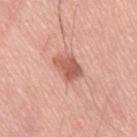Q: Was this lesion biopsied?
A: total-body-photography surveillance lesion; no biopsy
Q: What is the lesion's diameter?
A: about 3.5 mm
Q: What lighting was used for the tile?
A: white-light illumination
Q: How was this image acquired?
A: ~15 mm crop, total-body skin-cancer survey
Q: Where on the body is the lesion?
A: the right thigh
Q: What are the patient's age and sex?
A: male, aged 68 to 72